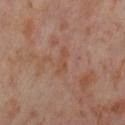image:
  source: total-body photography crop
  field_of_view_mm: 15
site: left thigh
patient:
  sex: female
  age_approx: 55
lesion_size:
  long_diameter_mm_approx: 3.5
automated_metrics:
  area_mm2_approx: 4.0
  eccentricity: 0.9
  shape_asymmetry: 0.25